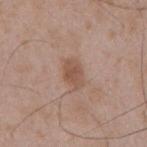Impression: No biopsy was performed on this lesion — it was imaged during a full skin examination and was not determined to be concerning. Background: From the mid back. Measured at roughly 3.5 mm in maximum diameter. A male patient, aged 48 to 52. A 15 mm close-up tile from a total-body photography series done for melanoma screening. Automated image analysis of the tile measured a border-irregularity index near 2.5/10, internal color variation of about 2 on a 0–10 scale, and radial color variation of about 0.5. And it measured an automated nevus-likeness rating near 25 out of 100 and a lesion-detection confidence of about 100/100. Captured under white-light illumination.